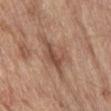Impression:
Imaged during a routine full-body skin examination; the lesion was not biopsied and no histopathology is available.
Image and clinical context:
From the front of the torso. Imaged with white-light lighting. Cropped from a total-body skin-imaging series; the visible field is about 15 mm. A male subject, in their 70s.